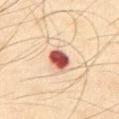This lesion was catalogued during total-body skin photography and was not selected for biopsy.
The lesion is located on the abdomen.
The patient is a male aged approximately 65.
Cropped from a total-body skin-imaging series; the visible field is about 15 mm.